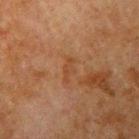Automated tile analysis of the lesion measured a footprint of about 3.5 mm² and an outline eccentricity of about 0.9 (0 = round, 1 = elongated). And it measured a mean CIELAB color near L≈36 a*≈18 b*≈30, about 4 CIELAB-L* units darker than the surrounding skin, and a lesion-to-skin contrast of about 5 (normalized; higher = more distinct).
Located on the left upper arm.
A male subject, about 80 years old.
A close-up tile cropped from a whole-body skin photograph, about 15 mm across.
Measured at roughly 3 mm in maximum diameter.
Captured under cross-polarized illumination.